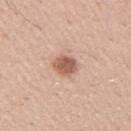  biopsy_status: not biopsied; imaged during a skin examination
  image:
    source: total-body photography crop
    field_of_view_mm: 15
  lighting: white-light
  patient:
    sex: male
    age_approx: 30
  site: right upper arm
  lesion_size:
    long_diameter_mm_approx: 3.0